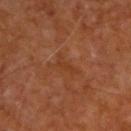| key | value |
|---|---|
| follow-up | imaged on a skin check; not biopsied |
| location | the upper back |
| illumination | cross-polarized |
| patient | male, roughly 65 years of age |
| acquisition | ~15 mm crop, total-body skin-cancer survey |